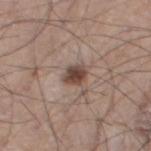| key | value |
|---|---|
| notes | catalogued during a skin exam; not biopsied |
| image | total-body-photography crop, ~15 mm field of view |
| illumination | white-light |
| location | the left thigh |
| lesion size | about 2.5 mm |
| patient | male, approximately 75 years of age |